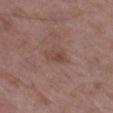No biopsy was performed on this lesion — it was imaged during a full skin examination and was not determined to be concerning.
The lesion's longest dimension is about 3 mm.
A close-up tile cropped from a whole-body skin photograph, about 15 mm across.
A male patient, aged 48 to 52.
Located on the leg.